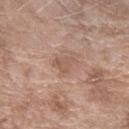Impression:
No biopsy was performed on this lesion — it was imaged during a full skin examination and was not determined to be concerning.
Acquisition and patient details:
A lesion tile, about 15 mm wide, cut from a 3D total-body photograph. A female patient, aged 73–77. The lesion's longest dimension is about 3 mm. This is a white-light tile. The total-body-photography lesion software estimated an average lesion color of about L≈55 a*≈19 b*≈27 (CIELAB), roughly 7 lightness units darker than nearby skin, and a normalized lesion–skin contrast near 5. The analysis additionally found a border-irregularity rating of about 4/10, a color-variation rating of about 2/10, and peripheral color asymmetry of about 0.5. On the right forearm.